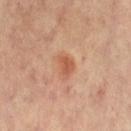notes=no biopsy performed (imaged during a skin exam); subject=female, about 65 years old; acquisition=~15 mm crop, total-body skin-cancer survey; location=the abdomen; tile lighting=cross-polarized.